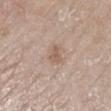Part of a total-body skin-imaging series; this lesion was reviewed on a skin check and was not flagged for biopsy. From the right lower leg. This image is a 15 mm lesion crop taken from a total-body photograph. A female subject aged approximately 65. About 2.5 mm across.